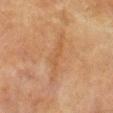This lesion was catalogued during total-body skin photography and was not selected for biopsy. Measured at roughly 6.5 mm in maximum diameter. The tile uses cross-polarized illumination. A 15 mm crop from a total-body photograph taken for skin-cancer surveillance. A male subject, roughly 60 years of age. On the leg.